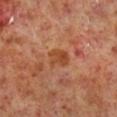Captured during whole-body skin photography for melanoma surveillance; the lesion was not biopsied. A male patient about 60 years old. Longest diameter approximately 3 mm. Located on the right lower leg. The tile uses cross-polarized illumination. A close-up tile cropped from a whole-body skin photograph, about 15 mm across.